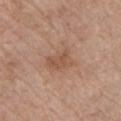biopsy status — catalogued during a skin exam; not biopsied
location — the front of the torso
acquisition — 15 mm crop, total-body photography
image-analysis metrics — roughly 8 lightness units darker than nearby skin and a lesion-to-skin contrast of about 5.5 (normalized; higher = more distinct); a border-irregularity rating of about 3.5/10 and a peripheral color-asymmetry measure near 1; an automated nevus-likeness rating near 0 out of 100 and a lesion-detection confidence of about 100/100
illumination — white-light illumination
patient — female, approximately 65 years of age